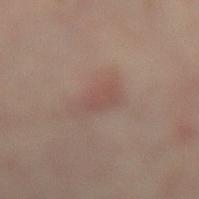Clinical impression: The lesion was photographed on a routine skin check and not biopsied; there is no pathology result. Context: The tile uses cross-polarized illumination. The lesion's longest dimension is about 4.5 mm. On the left lower leg. Automated image analysis of the tile measured an area of roughly 7 mm². It also reported border irregularity of about 4 on a 0–10 scale, a within-lesion color-variation index near 2/10, and peripheral color asymmetry of about 0.5. A 15 mm crop from a total-body photograph taken for skin-cancer surveillance. A female subject aged 33 to 37.